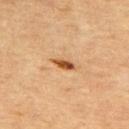Notes:
- biopsy status: catalogued during a skin exam; not biopsied
- site: the upper back
- imaging modality: 15 mm crop, total-body photography
- lighting: cross-polarized illumination
- patient: male, in their mid- to late 60s
- diameter: ≈3 mm
- automated lesion analysis: a lesion area of about 3 mm², an outline eccentricity of about 0.9 (0 = round, 1 = elongated), and two-axis asymmetry of about 0.25; a lesion–skin lightness drop of about 14 and a normalized lesion–skin contrast near 10.5; a color-variation rating of about 3.5/10 and radial color variation of about 1